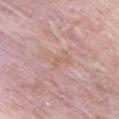Case summary:
• subject — male, aged around 75
• image — ~15 mm tile from a whole-body skin photo
• location — the chest
• tile lighting — white-light